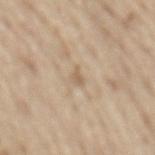An algorithmic analysis of the crop reported a border-irregularity rating of about 4.5/10 and radial color variation of about 0. A roughly 15 mm field-of-view crop from a total-body skin photograph. Approximately 2.5 mm at its widest. Located on the mid back. Imaged with white-light lighting. A male subject, aged approximately 70.